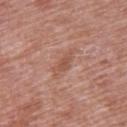Clinical impression:
Part of a total-body skin-imaging series; this lesion was reviewed on a skin check and was not flagged for biopsy.
Clinical summary:
A female subject aged 58 to 62. Automated tile analysis of the lesion measured a lesion area of about 3 mm², a shape eccentricity near 0.85, and a symmetry-axis asymmetry near 0.45. On the back. A 15 mm close-up extracted from a 3D total-body photography capture.